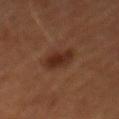– workup · no biopsy performed (imaged during a skin exam)
– size · ≈3.5 mm
– location · the mid back
– acquisition · total-body-photography crop, ~15 mm field of view
– subject · male, about 65 years old
– tile lighting · cross-polarized illumination